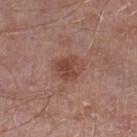Part of a total-body skin-imaging series; this lesion was reviewed on a skin check and was not flagged for biopsy.
Imaged with white-light lighting.
On the right lower leg.
A male patient, aged around 55.
A close-up tile cropped from a whole-body skin photograph, about 15 mm across.
Automated image analysis of the tile measured an area of roughly 8 mm² and a shape-asymmetry score of about 0.25 (0 = symmetric). It also reported a border-irregularity index near 2.5/10, a color-variation rating of about 4/10, and a peripheral color-asymmetry measure near 1.5. The analysis additionally found an automated nevus-likeness rating near 15 out of 100 and lesion-presence confidence of about 100/100.